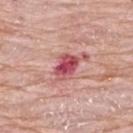Notes:
- workup: no biopsy performed (imaged during a skin exam)